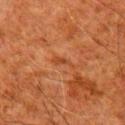| field | value |
|---|---|
| anatomic site | the right upper arm |
| TBP lesion metrics | an outline eccentricity of about 0.9 (0 = round, 1 = elongated) and a symmetry-axis asymmetry near 0.5; border irregularity of about 5.5 on a 0–10 scale, a within-lesion color-variation index near 0/10, and a peripheral color-asymmetry measure near 0 |
| diameter | ~3 mm (longest diameter) |
| patient | male, in their 80s |
| illumination | cross-polarized illumination |
| acquisition | 15 mm crop, total-body photography |